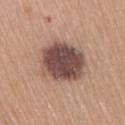{"biopsy_status": "not biopsied; imaged during a skin examination", "patient": {"sex": "female", "age_approx": 65}, "image": {"source": "total-body photography crop", "field_of_view_mm": 15}, "site": "right upper arm", "lesion_size": {"long_diameter_mm_approx": 5.5}, "lighting": "white-light"}